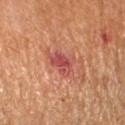Captured during whole-body skin photography for melanoma surveillance; the lesion was not biopsied.
Automated image analysis of the tile measured an eccentricity of roughly 0.65 and two-axis asymmetry of about 0.4. It also reported a lesion color around L≈49 a*≈31 b*≈27 in CIELAB, about 9 CIELAB-L* units darker than the surrounding skin, and a lesion-to-skin contrast of about 8 (normalized; higher = more distinct). And it measured a color-variation rating of about 5/10. The analysis additionally found an automated nevus-likeness rating near 5 out of 100 and lesion-presence confidence of about 100/100.
A 15 mm close-up tile from a total-body photography series done for melanoma screening.
Captured under cross-polarized illumination.
On the left arm.
A female patient aged approximately 65.
Longest diameter approximately 3.5 mm.